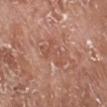Part of a total-body skin-imaging series; this lesion was reviewed on a skin check and was not flagged for biopsy.
The patient is a male aged 73 to 77.
Located on the right lower leg.
A region of skin cropped from a whole-body photographic capture, roughly 15 mm wide.
An algorithmic analysis of the crop reported a footprint of about 7 mm². The analysis additionally found roughly 6 lightness units darker than nearby skin and a normalized lesion–skin contrast near 4.5. And it measured a within-lesion color-variation index near 3/10 and a peripheral color-asymmetry measure near 1.
Imaged with white-light lighting.